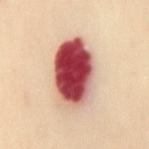Imaged during a routine full-body skin examination; the lesion was not biopsied and no histopathology is available. A 15 mm close-up extracted from a 3D total-body photography capture. On the mid back. Imaged with cross-polarized lighting. A female patient, aged 53 to 57. Measured at roughly 7.5 mm in maximum diameter. The lesion-visualizer software estimated an average lesion color of about L≈54 a*≈33 b*≈26 (CIELAB) and roughly 28 lightness units darker than nearby skin. The software also gave border irregularity of about 1 on a 0–10 scale, a color-variation rating of about 10/10, and radial color variation of about 5.5.